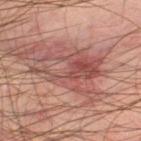A close-up tile cropped from a whole-body skin photograph, about 15 mm across. Captured under cross-polarized illumination. The lesion is located on the left thigh. A male subject aged 43 to 47. Approximately 8 mm at its widest.